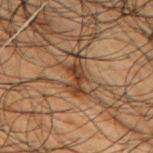Part of a total-body skin-imaging series; this lesion was reviewed on a skin check and was not flagged for biopsy. The patient is a male aged approximately 50. The lesion's longest dimension is about 4.5 mm. Cropped from a total-body skin-imaging series; the visible field is about 15 mm. From the right upper arm. Automated tile analysis of the lesion measured an average lesion color of about L≈30 a*≈16 b*≈25 (CIELAB), roughly 10 lightness units darker than nearby skin, and a lesion-to-skin contrast of about 9.5 (normalized; higher = more distinct). It also reported a border-irregularity index near 7/10 and a within-lesion color-variation index near 1.5/10. The analysis additionally found a nevus-likeness score of about 15/100 and a lesion-detection confidence of about 60/100. The tile uses cross-polarized illumination.